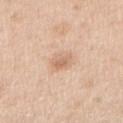Recorded during total-body skin imaging; not selected for excision or biopsy. Located on the right upper arm. Cropped from a whole-body photographic skin survey; the tile spans about 15 mm. The patient is a male aged 48 to 52.